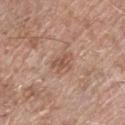Clinical summary:
A male subject, aged 63 to 67. This is a white-light tile. A 15 mm close-up extracted from a 3D total-body photography capture. The lesion is located on the chest.